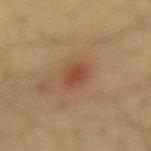Clinical impression:
The lesion was photographed on a routine skin check and not biopsied; there is no pathology result.
Context:
The lesion is on the lower back. A male subject, about 65 years old. A 15 mm close-up tile from a total-body photography series done for melanoma screening. Captured under cross-polarized illumination. Automated image analysis of the tile measured lesion-presence confidence of about 100/100.